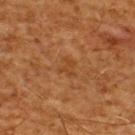Impression: Part of a total-body skin-imaging series; this lesion was reviewed on a skin check and was not flagged for biopsy. Image and clinical context: The total-body-photography lesion software estimated a lesion area of about 4 mm², an eccentricity of roughly 0.6, and two-axis asymmetry of about 0.4. And it measured a mean CIELAB color near L≈36 a*≈20 b*≈33, about 5 CIELAB-L* units darker than the surrounding skin, and a lesion-to-skin contrast of about 4.5 (normalized; higher = more distinct). It also reported a lesion-detection confidence of about 100/100. A 15 mm close-up tile from a total-body photography series done for melanoma screening. The tile uses cross-polarized illumination. The lesion's longest dimension is about 2.5 mm. A male subject, aged approximately 60. Located on the upper back.